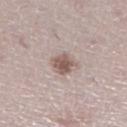Image and clinical context: A region of skin cropped from a whole-body photographic capture, roughly 15 mm wide. From the right lower leg. A female subject, about 50 years old.